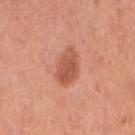Clinical impression: Part of a total-body skin-imaging series; this lesion was reviewed on a skin check and was not flagged for biopsy. Context: The lesion is on the right upper arm. The recorded lesion diameter is about 4.5 mm. A 15 mm close-up extracted from a 3D total-body photography capture. This is a white-light tile. Automated tile analysis of the lesion measured a lesion area of about 9 mm², an eccentricity of roughly 0.75, and a symmetry-axis asymmetry near 0.2. And it measured a border-irregularity index near 2/10 and a peripheral color-asymmetry measure near 1. The software also gave an automated nevus-likeness rating near 90 out of 100 and a detector confidence of about 100 out of 100 that the crop contains a lesion. A female subject aged 33 to 37.